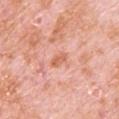Notes:
• biopsy status: total-body-photography surveillance lesion; no biopsy
• image source: 15 mm crop, total-body photography
• lesion size: about 2.5 mm
• anatomic site: the upper back
• subject: male, aged 78–82